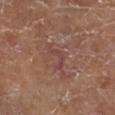Impression:
Imaged during a routine full-body skin examination; the lesion was not biopsied and no histopathology is available.
Background:
An algorithmic analysis of the crop reported a normalized border contrast of about 5. On the right lower leg. About 3.5 mm across. A 15 mm close-up tile from a total-body photography series done for melanoma screening. A female patient aged 73 to 77.